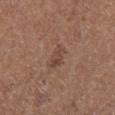- biopsy status: total-body-photography surveillance lesion; no biopsy
- imaging modality: total-body-photography crop, ~15 mm field of view
- subject: male, aged around 75
- lesion size: about 3 mm
- site: the right lower leg
- automated metrics: an eccentricity of roughly 0.75; a nevus-likeness score of about 0/100 and lesion-presence confidence of about 100/100